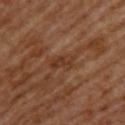Recorded during total-body skin imaging; not selected for excision or biopsy.
Cropped from a whole-body photographic skin survey; the tile spans about 15 mm.
The lesion is located on the upper back.
A female patient, in their mid- to late 60s.